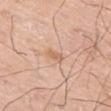Context:
This is a white-light tile. Measured at roughly 2.5 mm in maximum diameter. On the left thigh. The subject is a male aged 58 to 62. A close-up tile cropped from a whole-body skin photograph, about 15 mm across.